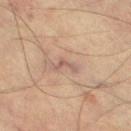| field | value |
|---|---|
| follow-up | total-body-photography surveillance lesion; no biopsy |
| image source | 15 mm crop, total-body photography |
| patient | male, in their mid- to late 70s |
| illumination | cross-polarized |
| site | the left thigh |